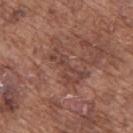Impression:
The lesion was photographed on a routine skin check and not biopsied; there is no pathology result.
Clinical summary:
This is a white-light tile. The subject is a male roughly 75 years of age. Located on the upper back. About 6 mm across. Automated tile analysis of the lesion measured a border-irregularity index near 8/10, a color-variation rating of about 3/10, and peripheral color asymmetry of about 1. A close-up tile cropped from a whole-body skin photograph, about 15 mm across.